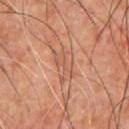A male subject approximately 60 years of age.
On the front of the torso.
Approximately 4 mm at its widest.
Cropped from a whole-body photographic skin survey; the tile spans about 15 mm.
This is a cross-polarized tile.